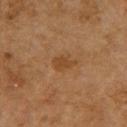  biopsy_status: not biopsied; imaged during a skin examination
  patient:
    sex: female
    age_approx: 60
  site: upper back
  image:
    source: total-body photography crop
    field_of_view_mm: 15